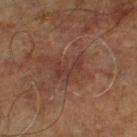{
  "biopsy_status": "not biopsied; imaged during a skin examination",
  "site": "leg",
  "patient": {
    "sex": "male",
    "age_approx": 70
  },
  "lighting": "cross-polarized",
  "image": {
    "source": "total-body photography crop",
    "field_of_view_mm": 15
  },
  "lesion_size": {
    "long_diameter_mm_approx": 3.0
  }
}